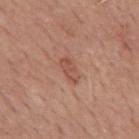– workup: total-body-photography surveillance lesion; no biopsy
– automated metrics: an automated nevus-likeness rating near 0 out of 100 and a detector confidence of about 100 out of 100 that the crop contains a lesion
– acquisition: ~15 mm tile from a whole-body skin photo
– lighting: white-light
– body site: the mid back
– patient: male, roughly 50 years of age